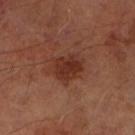This lesion was catalogued during total-body skin photography and was not selected for biopsy. This image is a 15 mm lesion crop taken from a total-body photograph. A male subject, roughly 70 years of age. An algorithmic analysis of the crop reported an area of roughly 9.5 mm² and two-axis asymmetry of about 0.25. About 4 mm across. The lesion is located on the arm. Imaged with cross-polarized lighting.